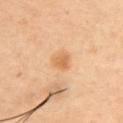Findings:
* notes: total-body-photography surveillance lesion; no biopsy
* image: total-body-photography crop, ~15 mm field of view
* subject: male, roughly 40 years of age
* diameter: about 3 mm
* illumination: cross-polarized illumination
* site: the upper back
* automated metrics: a lesion area of about 6 mm² and a shape-asymmetry score of about 0.15 (0 = symmetric); a mean CIELAB color near L≈66 a*≈20 b*≈40, roughly 8 lightness units darker than nearby skin, and a normalized lesion–skin contrast near 6; a within-lesion color-variation index near 3/10 and peripheral color asymmetry of about 1; a classifier nevus-likeness of about 55/100 and a lesion-detection confidence of about 100/100